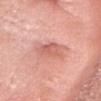Impression:
The lesion was tiled from a total-body skin photograph and was not biopsied.
Image and clinical context:
A close-up tile cropped from a whole-body skin photograph, about 15 mm across. Located on the head or neck. A female subject aged approximately 70.